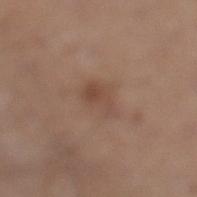| field | value |
|---|---|
| patient | male, aged 63–67 |
| imaging modality | total-body-photography crop, ~15 mm field of view |
| location | the left lower leg |